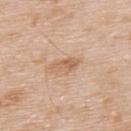| feature | finding |
|---|---|
| notes | catalogued during a skin exam; not biopsied |